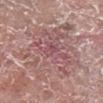Assessment:
Part of a total-body skin-imaging series; this lesion was reviewed on a skin check and was not flagged for biopsy.
Context:
This image is a 15 mm lesion crop taken from a total-body photograph. The lesion is on the right lower leg. Automated tile analysis of the lesion measured a mean CIELAB color near L≈55 a*≈21 b*≈20, about 7 CIELAB-L* units darker than the surrounding skin, and a normalized lesion–skin contrast near 5.5. A male patient, approximately 75 years of age. The tile uses white-light illumination.